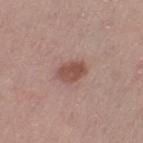| key | value |
|---|---|
| follow-up | catalogued during a skin exam; not biopsied |
| acquisition | 15 mm crop, total-body photography |
| size | ≈3.5 mm |
| tile lighting | white-light |
| body site | the left thigh |
| patient | female, about 50 years old |